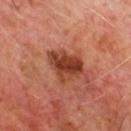<record>
<biopsy_status>not biopsied; imaged during a skin examination</biopsy_status>
<image>
  <source>total-body photography crop</source>
  <field_of_view_mm>15</field_of_view_mm>
</image>
<lighting>cross-polarized</lighting>
<automated_metrics>
  <shape_asymmetry>0.35</shape_asymmetry>
  <cielab_L>42</cielab_L>
  <cielab_a>29</cielab_a>
  <cielab_b>34</cielab_b>
  <vs_skin_darker_L>13.0</vs_skin_darker_L>
  <lesion_detection_confidence_0_100>100</lesion_detection_confidence_0_100>
</automated_metrics>
<site>chest</site>
<patient>
  <sex>male</sex>
  <age_approx>65</age_approx>
</patient>
</record>